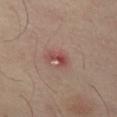Clinical impression:
The lesion was photographed on a routine skin check and not biopsied; there is no pathology result.
Clinical summary:
On the leg. About 3 mm across. The lesion-visualizer software estimated an average lesion color of about L≈48 a*≈27 b*≈24 (CIELAB), roughly 9 lightness units darker than nearby skin, and a normalized lesion–skin contrast near 7. A lesion tile, about 15 mm wide, cut from a 3D total-body photograph. A female subject, roughly 55 years of age.